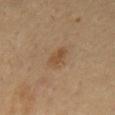notes: total-body-photography surveillance lesion; no biopsy | subject: male, about 55 years old | lesion diameter: ≈3 mm | site: the chest | image: total-body-photography crop, ~15 mm field of view | image-analysis metrics: a footprint of about 4.5 mm² and an eccentricity of roughly 0.85; a border-irregularity rating of about 3/10, a color-variation rating of about 2.5/10, and peripheral color asymmetry of about 1; lesion-presence confidence of about 100/100.